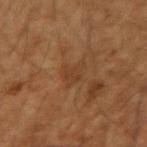Imaged during a routine full-body skin examination; the lesion was not biopsied and no histopathology is available. An algorithmic analysis of the crop reported a lesion area of about 5 mm², an eccentricity of roughly 0.55, and a symmetry-axis asymmetry near 0.4. And it measured a mean CIELAB color near L≈37 a*≈19 b*≈31 and roughly 5 lightness units darker than nearby skin. The analysis additionally found a detector confidence of about 100 out of 100 that the crop contains a lesion. The lesion is on the right upper arm. Imaged with cross-polarized lighting. A region of skin cropped from a whole-body photographic capture, roughly 15 mm wide. A male patient in their mid-50s.